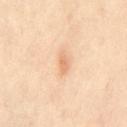No biopsy was performed on this lesion — it was imaged during a full skin examination and was not determined to be concerning. This is a cross-polarized tile. Approximately 3 mm at its widest. Located on the right leg. The patient is a female aged 38–42. A close-up tile cropped from a whole-body skin photograph, about 15 mm across. The total-body-photography lesion software estimated a mean CIELAB color near L≈74 a*≈21 b*≈38, a lesion–skin lightness drop of about 9, and a normalized lesion–skin contrast near 6. The analysis additionally found a classifier nevus-likeness of about 5/100 and lesion-presence confidence of about 100/100.